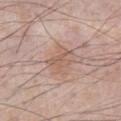Approximately 3 mm at its widest. The lesion is located on the chest. Cropped from a whole-body photographic skin survey; the tile spans about 15 mm. A male patient aged approximately 70.